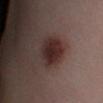follow-up = total-body-photography surveillance lesion; no biopsy
image-analysis metrics = an area of roughly 13 mm² and an eccentricity of roughly 0.35; a border-irregularity rating of about 1.5/10, internal color variation of about 4.5 on a 0–10 scale, and radial color variation of about 1.5
patient = male, aged approximately 40
image source = 15 mm crop, total-body photography
lighting = cross-polarized illumination
size = about 4 mm
location = the left lower leg